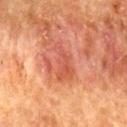Assessment:
This lesion was catalogued during total-body skin photography and was not selected for biopsy.
Acquisition and patient details:
The recorded lesion diameter is about 4.5 mm. The lesion is located on the mid back. The tile uses cross-polarized illumination. A lesion tile, about 15 mm wide, cut from a 3D total-body photograph. A male subject aged 48 to 52.